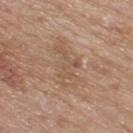workup = catalogued during a skin exam; not biopsied | patient = male, aged approximately 75 | image = 15 mm crop, total-body photography | body site = the upper back | lesion size = about 6 mm | tile lighting = white-light illumination.